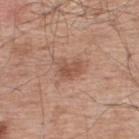Q: Was a biopsy performed?
A: imaged on a skin check; not biopsied
Q: What are the patient's age and sex?
A: male, aged 53–57
Q: What did automated image analysis measure?
A: a lesion area of about 5.5 mm², a shape eccentricity near 0.75, and a symmetry-axis asymmetry near 0.3; a mean CIELAB color near L≈53 a*≈22 b*≈29, about 9 CIELAB-L* units darker than the surrounding skin, and a lesion-to-skin contrast of about 6.5 (normalized; higher = more distinct); a border-irregularity index near 3/10, a within-lesion color-variation index near 2/10, and a peripheral color-asymmetry measure near 1
Q: What lighting was used for the tile?
A: white-light illumination
Q: How large is the lesion?
A: ≈3 mm
Q: Where on the body is the lesion?
A: the upper back
Q: What is the imaging modality?
A: ~15 mm tile from a whole-body skin photo